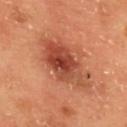Part of a total-body skin-imaging series; this lesion was reviewed on a skin check and was not flagged for biopsy.
The lesion's longest dimension is about 7 mm.
Automated image analysis of the tile measured an area of roughly 18 mm², an eccentricity of roughly 0.85, and a shape-asymmetry score of about 0.3 (0 = symmetric). The software also gave an automated nevus-likeness rating near 65 out of 100 and a detector confidence of about 100 out of 100 that the crop contains a lesion.
A 15 mm crop from a total-body photograph taken for skin-cancer surveillance.
This is a cross-polarized tile.
A female patient, about 50 years old.
The lesion is located on the upper back.